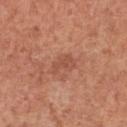Recorded during total-body skin imaging; not selected for excision or biopsy.
Measured at roughly 2.5 mm in maximum diameter.
A male patient aged 63–67.
This is a white-light tile.
Automated image analysis of the tile measured an average lesion color of about L≈51 a*≈26 b*≈31 (CIELAB), a lesion–skin lightness drop of about 7, and a lesion-to-skin contrast of about 5.5 (normalized; higher = more distinct).
On the chest.
A 15 mm crop from a total-body photograph taken for skin-cancer surveillance.